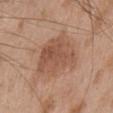Captured during whole-body skin photography for melanoma surveillance; the lesion was not biopsied.
A roughly 15 mm field-of-view crop from a total-body skin photograph.
On the chest.
About 6.5 mm across.
This is a white-light tile.
A male patient, aged 63 to 67.